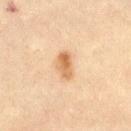{"biopsy_status": "not biopsied; imaged during a skin examination", "patient": {"sex": "female", "age_approx": 45}, "site": "leg", "lesion_size": {"long_diameter_mm_approx": 3.5}, "image": {"source": "total-body photography crop", "field_of_view_mm": 15}}